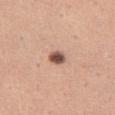Case summary:
- biopsy status — imaged on a skin check; not biopsied